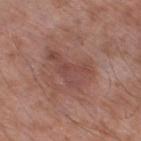Imaged during a routine full-body skin examination; the lesion was not biopsied and no histopathology is available.
A male patient aged 53–57.
Approximately 5.5 mm at its widest.
Cropped from a total-body skin-imaging series; the visible field is about 15 mm.
The lesion is located on the leg.
This is a white-light tile.